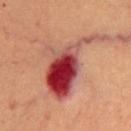The lesion was tiled from a total-body skin photograph and was not biopsied. Longest diameter approximately 9.5 mm. Located on the mid back. A male patient approximately 65 years of age. A lesion tile, about 15 mm wide, cut from a 3D total-body photograph. Captured under cross-polarized illumination.